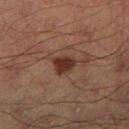Clinical impression: Captured during whole-body skin photography for melanoma surveillance; the lesion was not biopsied.